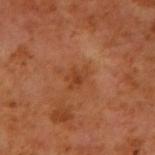A male patient, about 60 years old.
A close-up tile cropped from a whole-body skin photograph, about 15 mm across.
The lesion is on the right upper arm.